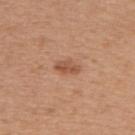No biopsy was performed on this lesion — it was imaged during a full skin examination and was not determined to be concerning. A 15 mm close-up tile from a total-body photography series done for melanoma screening. The lesion is located on the upper back. A female subject, approximately 55 years of age.